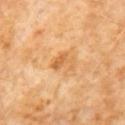Part of a total-body skin-imaging series; this lesion was reviewed on a skin check and was not flagged for biopsy.
A region of skin cropped from a whole-body photographic capture, roughly 15 mm wide.
The lesion's longest dimension is about 3 mm.
On the abdomen.
This is a cross-polarized tile.
A male subject, roughly 60 years of age.